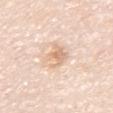notes = no biopsy performed (imaged during a skin exam).